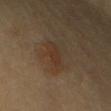Impression: Recorded during total-body skin imaging; not selected for excision or biopsy. Context: The lesion is on the arm. Longest diameter approximately 2.5 mm. The subject is aged around 60. Captured under cross-polarized illumination. This image is a 15 mm lesion crop taken from a total-body photograph. The total-body-photography lesion software estimated a shape eccentricity near 0.75 and a shape-asymmetry score of about 0.35 (0 = symmetric). The analysis additionally found about 5 CIELAB-L* units darker than the surrounding skin and a lesion-to-skin contrast of about 5.5 (normalized; higher = more distinct).